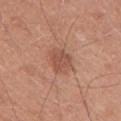Q: What is the lesion's diameter?
A: ≈3 mm
Q: Illumination type?
A: white-light illumination
Q: How was this image acquired?
A: total-body-photography crop, ~15 mm field of view
Q: What are the patient's age and sex?
A: male, aged approximately 30
Q: What is the anatomic site?
A: the right upper arm